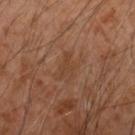Recorded during total-body skin imaging; not selected for excision or biopsy. A region of skin cropped from a whole-body photographic capture, roughly 15 mm wide. A male patient, aged approximately 55. Measured at roughly 3 mm in maximum diameter. The lesion is on the arm.